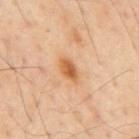Clinical impression:
Part of a total-body skin-imaging series; this lesion was reviewed on a skin check and was not flagged for biopsy.
Clinical summary:
The total-body-photography lesion software estimated a lesion color around L≈62 a*≈24 b*≈41 in CIELAB, about 12 CIELAB-L* units darker than the surrounding skin, and a normalized border contrast of about 8.5. The lesion is on the mid back. About 3.5 mm across. The subject is a male about 55 years old. Imaged with cross-polarized lighting. Cropped from a whole-body photographic skin survey; the tile spans about 15 mm.